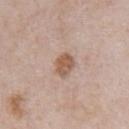biopsy status: no biopsy performed (imaged during a skin exam)
acquisition: ~15 mm crop, total-body skin-cancer survey
illumination: white-light illumination
automated metrics: a footprint of about 5.5 mm², an outline eccentricity of about 0.6 (0 = round, 1 = elongated), and a symmetry-axis asymmetry near 0.2; a mean CIELAB color near L≈56 a*≈19 b*≈29, a lesion–skin lightness drop of about 11, and a normalized lesion–skin contrast near 8; a within-lesion color-variation index near 2/10 and a peripheral color-asymmetry measure near 0.5; a classifier nevus-likeness of about 45/100 and a lesion-detection confidence of about 100/100
subject: male, aged approximately 80
location: the front of the torso